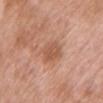Assessment: Recorded during total-body skin imaging; not selected for excision or biopsy. Background: Captured under white-light illumination. A female subject about 70 years old. On the chest. Approximately 3.5 mm at its widest. A 15 mm close-up extracted from a 3D total-body photography capture.